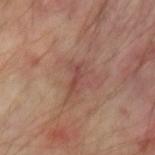Cropped from a total-body skin-imaging series; the visible field is about 15 mm.
Longest diameter approximately 3.5 mm.
This is a cross-polarized tile.
Located on the left forearm.
A patient aged around 55.